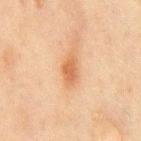illumination = cross-polarized | imaging modality = ~15 mm tile from a whole-body skin photo | subject = male, roughly 45 years of age | anatomic site = the front of the torso.